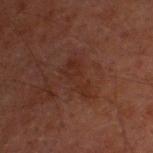TBP lesion metrics — an eccentricity of roughly 0.9 and two-axis asymmetry of about 0.5; a lesion color around L≈21 a*≈17 b*≈20 in CIELAB, about 4 CIELAB-L* units darker than the surrounding skin, and a lesion-to-skin contrast of about 5 (normalized; higher = more distinct); a border-irregularity index near 6.5/10 and a color-variation rating of about 1.5/10; a classifier nevus-likeness of about 0/100 and a lesion-detection confidence of about 100/100
location — the head or neck
diameter — ≈5 mm
patient — male, in their 50s
image — total-body-photography crop, ~15 mm field of view